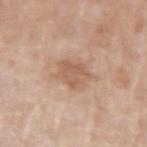Captured during whole-body skin photography for melanoma surveillance; the lesion was not biopsied. A close-up tile cropped from a whole-body skin photograph, about 15 mm across. About 3.5 mm across. On the left upper arm. A male patient, about 60 years old.